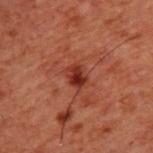{
  "biopsy_status": "not biopsied; imaged during a skin examination",
  "site": "upper back",
  "image": {
    "source": "total-body photography crop",
    "field_of_view_mm": 15
  },
  "patient": {
    "sex": "male",
    "age_approx": 60
  }
}